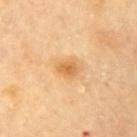Part of a total-body skin-imaging series; this lesion was reviewed on a skin check and was not flagged for biopsy. The total-body-photography lesion software estimated a footprint of about 4 mm², an outline eccentricity of about 0.65 (0 = round, 1 = elongated), and two-axis asymmetry of about 0.25. The software also gave a classifier nevus-likeness of about 55/100 and lesion-presence confidence of about 100/100. A close-up tile cropped from a whole-body skin photograph, about 15 mm across. About 2.5 mm across. The lesion is located on the left upper arm. A male subject in their mid- to late 80s or older. Imaged with cross-polarized lighting.